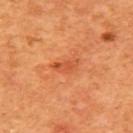Case summary:
* workup: imaged on a skin check; not biopsied
* anatomic site: the upper back
* illumination: cross-polarized illumination
* acquisition: ~15 mm crop, total-body skin-cancer survey
* patient: female, about 40 years old
* lesion diameter: ~3 mm (longest diameter)
* automated lesion analysis: a lesion color around L≈55 a*≈35 b*≈44 in CIELAB and about 9 CIELAB-L* units darker than the surrounding skin